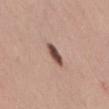Captured during whole-body skin photography for melanoma surveillance; the lesion was not biopsied. Automated image analysis of the tile measured a footprint of about 4 mm² and an outline eccentricity of about 0.85 (0 = round, 1 = elongated). The software also gave roughly 18 lightness units darker than nearby skin and a normalized border contrast of about 12. And it measured border irregularity of about 2 on a 0–10 scale. A roughly 15 mm field-of-view crop from a total-body skin photograph. Located on the lower back. This is a white-light tile. A female subject approximately 35 years of age. The recorded lesion diameter is about 3 mm.